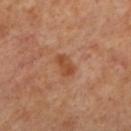Recorded during total-body skin imaging; not selected for excision or biopsy.
The lesion's longest dimension is about 3 mm.
A lesion tile, about 15 mm wide, cut from a 3D total-body photograph.
The total-body-photography lesion software estimated a shape eccentricity near 0.85 and a symmetry-axis asymmetry near 0.25. It also reported a lesion color around L≈49 a*≈25 b*≈36 in CIELAB, roughly 9 lightness units darker than nearby skin, and a normalized lesion–skin contrast near 7.5. The analysis additionally found a classifier nevus-likeness of about 10/100 and a lesion-detection confidence of about 100/100.
On the left lower leg.
The tile uses cross-polarized illumination.
The subject is a female about 55 years old.